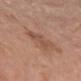Notes:
– workup: total-body-photography surveillance lesion; no biopsy
– site: the chest
– subject: female, in their mid- to late 80s or older
– lighting: white-light
– acquisition: ~15 mm tile from a whole-body skin photo